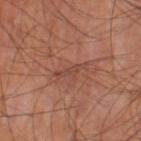Captured during whole-body skin photography for melanoma surveillance; the lesion was not biopsied.
A roughly 15 mm field-of-view crop from a total-body skin photograph.
From the left thigh.
The subject is a male aged 63–67.